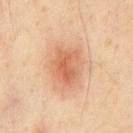| feature | finding |
|---|---|
| subject | male, in their mid- to late 50s |
| size | ≈5 mm |
| TBP lesion metrics | a lesion area of about 14 mm², an eccentricity of roughly 0.65, and two-axis asymmetry of about 0.2; a border-irregularity index near 2/10, internal color variation of about 5.5 on a 0–10 scale, and radial color variation of about 2 |
| illumination | cross-polarized |
| site | the chest |
| image source | ~15 mm tile from a whole-body skin photo |